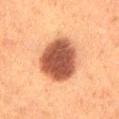<case>
  <biopsy_status>not biopsied; imaged during a skin examination</biopsy_status>
  <patient>
    <sex>female</sex>
    <age_approx>50</age_approx>
  </patient>
  <site>lower back</site>
  <lesion_size>
    <long_diameter_mm_approx>6.0</long_diameter_mm_approx>
  </lesion_size>
  <image>
    <source>total-body photography crop</source>
    <field_of_view_mm>15</field_of_view_mm>
  </image>
</case>